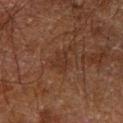Assessment:
Recorded during total-body skin imaging; not selected for excision or biopsy.
Acquisition and patient details:
The subject is a male in their 60s. Imaged with cross-polarized lighting. From the arm. Longest diameter approximately 2.5 mm. A 15 mm close-up extracted from a 3D total-body photography capture.